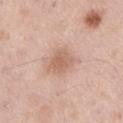| feature | finding |
|---|---|
| biopsy status | total-body-photography surveillance lesion; no biopsy |